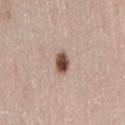{
  "patient": {
    "sex": "female",
    "age_approx": 50
  },
  "image": {
    "source": "total-body photography crop",
    "field_of_view_mm": 15
  },
  "lesion_size": {
    "long_diameter_mm_approx": 2.5
  },
  "site": "back",
  "automated_metrics": {
    "cielab_L": 50,
    "cielab_a": 19,
    "cielab_b": 26,
    "vs_skin_darker_L": 19.0,
    "vs_skin_contrast_norm": 12.5,
    "lesion_detection_confidence_0_100": 100
  },
  "lighting": "white-light"
}